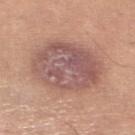The lesion was tiled from a total-body skin photograph and was not biopsied. A 15 mm close-up tile from a total-body photography series done for melanoma screening. On the leg. The tile uses white-light illumination. A female subject, in their mid-40s. The lesion's longest dimension is about 7.5 mm.